The lesion-visualizer software estimated an area of roughly 4.5 mm², an eccentricity of roughly 0.7, and two-axis asymmetry of about 0.25. And it measured an average lesion color of about L≈63 a*≈25 b*≈30 (CIELAB), a lesion–skin lightness drop of about 7, and a normalized border contrast of about 5.5; a female subject, roughly 40 years of age; the lesion is located on the right upper arm; a 15 mm close-up tile from a total-body photography series done for melanoma screening; about 2.5 mm across:
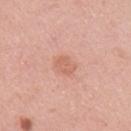The biopsy diagnosis was a dysplastic (Clark) nevus, classified as a benign lesion.